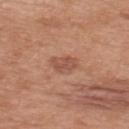The lesion was photographed on a routine skin check and not biopsied; there is no pathology result. This is a white-light tile. About 3 mm across. Located on the upper back. A close-up tile cropped from a whole-body skin photograph, about 15 mm across. The subject is a male roughly 70 years of age.